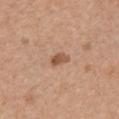The lesion was tiled from a total-body skin photograph and was not biopsied.
Imaged with white-light lighting.
About 2.5 mm across.
The subject is a female aged around 75.
A 15 mm close-up tile from a total-body photography series done for melanoma screening.
From the right upper arm.